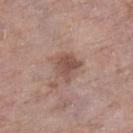– biopsy status · imaged on a skin check; not biopsied
– imaging modality · ~15 mm tile from a whole-body skin photo
– patient · female, aged 83 or older
– tile lighting · white-light
– diameter · ~3.5 mm (longest diameter)
– body site · the right lower leg